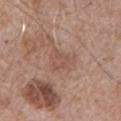Captured during whole-body skin photography for melanoma surveillance; the lesion was not biopsied. The total-body-photography lesion software estimated a symmetry-axis asymmetry near 0.45. And it measured a border-irregularity index near 4.5/10, a color-variation rating of about 2/10, and a peripheral color-asymmetry measure near 0.5. The software also gave lesion-presence confidence of about 100/100. Located on the chest. A male subject, aged around 65. A roughly 15 mm field-of-view crop from a total-body skin photograph. The lesion's longest dimension is about 3.5 mm.